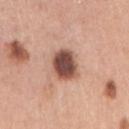<lesion>
<biopsy_status>not biopsied; imaged during a skin examination</biopsy_status>
<patient>
  <sex>female</sex>
  <age_approx>60</age_approx>
</patient>
<image>
  <source>total-body photography crop</source>
  <field_of_view_mm>15</field_of_view_mm>
</image>
<site>arm</site>
</lesion>